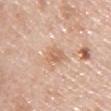biopsy status: total-body-photography surveillance lesion; no biopsy
lighting: white-light
subject: male, in their 50s
body site: the chest
automated metrics: an average lesion color of about L≈64 a*≈20 b*≈33 (CIELAB), a lesion–skin lightness drop of about 8, and a normalized border contrast of about 6; lesion-presence confidence of about 100/100
lesion diameter: ≈3 mm
image source: ~15 mm crop, total-body skin-cancer survey